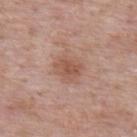Q: Was this lesion biopsied?
A: total-body-photography surveillance lesion; no biopsy
Q: How was this image acquired?
A: 15 mm crop, total-body photography
Q: What are the patient's age and sex?
A: male, about 55 years old
Q: Where on the body is the lesion?
A: the upper back
Q: How large is the lesion?
A: ~3 mm (longest diameter)
Q: How was the tile lit?
A: white-light illumination